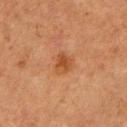This lesion was catalogued during total-body skin photography and was not selected for biopsy.
A male patient approximately 75 years of age.
The lesion is located on the left upper arm.
A 15 mm close-up tile from a total-body photography series done for melanoma screening.